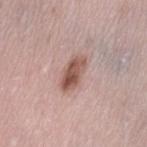Acquisition and patient details: The tile uses white-light illumination. Approximately 4.5 mm at its widest. The total-body-photography lesion software estimated an area of roughly 8 mm², an eccentricity of roughly 0.85, and a shape-asymmetry score of about 0.2 (0 = symmetric). And it measured roughly 13 lightness units darker than nearby skin and a normalized lesion–skin contrast near 9. And it measured a border-irregularity rating of about 2.5/10 and a color-variation rating of about 6.5/10. The analysis additionally found a nevus-likeness score of about 80/100. A female patient, aged approximately 40. This image is a 15 mm lesion crop taken from a total-body photograph. The lesion is on the left thigh.